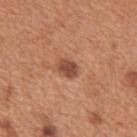Clinical impression: No biopsy was performed on this lesion — it was imaged during a full skin examination and was not determined to be concerning. Context: The recorded lesion diameter is about 2.5 mm. A roughly 15 mm field-of-view crop from a total-body skin photograph. A male patient aged 63–67. Automated tile analysis of the lesion measured a mean CIELAB color near L≈48 a*≈24 b*≈31, roughly 13 lightness units darker than nearby skin, and a normalized border contrast of about 9. It also reported a border-irregularity index near 1/10, internal color variation of about 2.5 on a 0–10 scale, and a peripheral color-asymmetry measure near 1. It also reported a detector confidence of about 100 out of 100 that the crop contains a lesion. Located on the back.